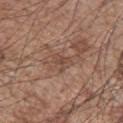| feature | finding |
|---|---|
| follow-up | imaged on a skin check; not biopsied |
| patient | male, aged approximately 65 |
| illumination | white-light illumination |
| site | the left upper arm |
| image-analysis metrics | a lesion color around L≈46 a*≈19 b*≈26 in CIELAB, roughly 7 lightness units darker than nearby skin, and a lesion-to-skin contrast of about 5.5 (normalized; higher = more distinct); a border-irregularity index near 6/10, a color-variation rating of about 2/10, and a peripheral color-asymmetry measure near 1; an automated nevus-likeness rating near 0 out of 100 and lesion-presence confidence of about 80/100 |
| acquisition | ~15 mm crop, total-body skin-cancer survey |
| diameter | about 2.5 mm |